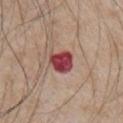Captured during whole-body skin photography for melanoma surveillance; the lesion was not biopsied. A lesion tile, about 15 mm wide, cut from a 3D total-body photograph. This is a white-light tile. On the chest. A male patient aged 63–67. Measured at roughly 3 mm in maximum diameter.